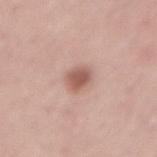Clinical impression: The lesion was photographed on a routine skin check and not biopsied; there is no pathology result. Context: A male subject, roughly 55 years of age. About 3 mm across. An algorithmic analysis of the crop reported an area of roughly 6 mm² and two-axis asymmetry of about 0.15. And it measured a detector confidence of about 100 out of 100 that the crop contains a lesion. The lesion is located on the lower back. This is a white-light tile. A region of skin cropped from a whole-body photographic capture, roughly 15 mm wide.